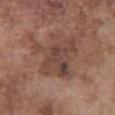Approximately 6 mm at its widest. A male patient in their mid-70s. From the abdomen. A region of skin cropped from a whole-body photographic capture, roughly 15 mm wide. Captured under white-light illumination.